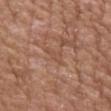The lesion was tiled from a total-body skin photograph and was not biopsied. The subject is a male in their mid- to late 60s. An algorithmic analysis of the crop reported border irregularity of about 5.5 on a 0–10 scale, a color-variation rating of about 0.5/10, and radial color variation of about 0. And it measured a nevus-likeness score of about 0/100. Cropped from a whole-body photographic skin survey; the tile spans about 15 mm. The lesion is on the arm. About 3 mm across. This is a white-light tile.